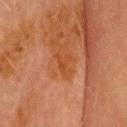Q: Was this lesion biopsied?
A: total-body-photography surveillance lesion; no biopsy
Q: What are the patient's age and sex?
A: male, approximately 70 years of age
Q: What kind of image is this?
A: 15 mm crop, total-body photography
Q: How large is the lesion?
A: about 2.5 mm
Q: What is the anatomic site?
A: the head or neck
Q: What lighting was used for the tile?
A: cross-polarized illumination